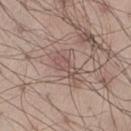notes: total-body-photography surveillance lesion; no biopsy | body site: the left thigh | diameter: ~3 mm (longest diameter) | image-analysis metrics: a lesion color around L≈52 a*≈18 b*≈22 in CIELAB, a lesion–skin lightness drop of about 7, and a lesion-to-skin contrast of about 5 (normalized; higher = more distinct); an automated nevus-likeness rating near 0 out of 100 | tile lighting: white-light | image source: 15 mm crop, total-body photography | subject: male, in their 30s.